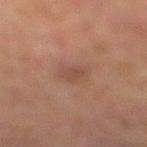{"biopsy_status": "not biopsied; imaged during a skin examination", "lighting": "cross-polarized", "patient": {"sex": "male", "age_approx": 55}, "automated_metrics": {"area_mm2_approx": 4.5, "cielab_L": 42, "cielab_a": 19, "cielab_b": 25, "vs_skin_darker_L": 6.0, "vs_skin_contrast_norm": 5.0, "color_variation_0_10": 1.0, "peripheral_color_asymmetry": 0.5, "nevus_likeness_0_100": 5}, "image": {"source": "total-body photography crop", "field_of_view_mm": 15}, "site": "right lower leg", "lesion_size": {"long_diameter_mm_approx": 2.5}}